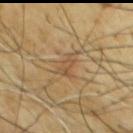biopsy_status: not biopsied; imaged during a skin examination
lighting: cross-polarized
site: chest
image:
  source: total-body photography crop
  field_of_view_mm: 15
patient:
  sex: male
  age_approx: 65